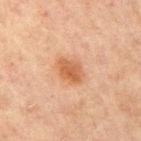Assessment:
This lesion was catalogued during total-body skin photography and was not selected for biopsy.
Acquisition and patient details:
The lesion is on the right upper arm. About 3.5 mm across. A 15 mm crop from a total-body photograph taken for skin-cancer surveillance. Automated tile analysis of the lesion measured an average lesion color of about L≈51 a*≈22 b*≈34 (CIELAB), roughly 10 lightness units darker than nearby skin, and a normalized lesion–skin contrast near 8. And it measured a border-irregularity index near 2/10. A male patient aged around 45.